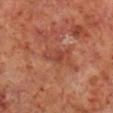Acquisition and patient details: A male patient aged 58 to 62. A close-up tile cropped from a whole-body skin photograph, about 15 mm across. The tile uses cross-polarized illumination. Automated tile analysis of the lesion measured an outline eccentricity of about 0.85 (0 = round, 1 = elongated) and a symmetry-axis asymmetry near 0.65. And it measured a border-irregularity rating of about 7/10, a within-lesion color-variation index near 0.5/10, and peripheral color asymmetry of about 0. The analysis additionally found an automated nevus-likeness rating near 0 out of 100 and lesion-presence confidence of about 95/100. On the right lower leg.